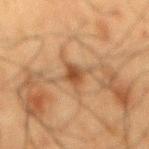workup=catalogued during a skin exam; not biopsied | subject=male, approximately 60 years of age | location=the mid back | acquisition=~15 mm crop, total-body skin-cancer survey.